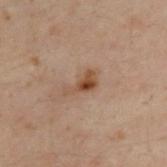This lesion was catalogued during total-body skin photography and was not selected for biopsy. Imaged with cross-polarized lighting. A male subject roughly 30 years of age. The lesion-visualizer software estimated a lesion area of about 4.5 mm² and an eccentricity of roughly 0.9. And it measured a nevus-likeness score of about 55/100 and lesion-presence confidence of about 100/100. The recorded lesion diameter is about 3.5 mm. Located on the upper back. A 15 mm close-up tile from a total-body photography series done for melanoma screening.